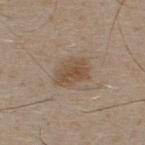The lesion was tiled from a total-body skin photograph and was not biopsied.
Approximately 4 mm at its widest.
On the upper back.
A male subject, approximately 30 years of age.
Cropped from a total-body skin-imaging series; the visible field is about 15 mm.
The tile uses white-light illumination.